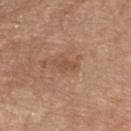| key | value |
|---|---|
| follow-up | total-body-photography surveillance lesion; no biopsy |
| patient | male, aged 78–82 |
| lesion size | ~3 mm (longest diameter) |
| TBP lesion metrics | a footprint of about 3.5 mm², an outline eccentricity of about 0.85 (0 = round, 1 = elongated), and two-axis asymmetry of about 0.3; a lesion color around L≈51 a*≈21 b*≈32 in CIELAB, roughly 7 lightness units darker than nearby skin, and a normalized border contrast of about 6; a classifier nevus-likeness of about 0/100 and a detector confidence of about 100 out of 100 that the crop contains a lesion |
| body site | the chest |
| acquisition | total-body-photography crop, ~15 mm field of view |
| illumination | white-light |